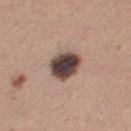workup = imaged on a skin check; not biopsied | location = the left thigh | patient = female, aged 28–32 | imaging modality = ~15 mm crop, total-body skin-cancer survey | lighting = white-light | diameter = ~4 mm (longest diameter) | automated metrics = a border-irregularity rating of about 1.5/10 and radial color variation of about 1.5.